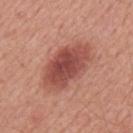This is a white-light tile.
A lesion tile, about 15 mm wide, cut from a 3D total-body photograph.
From the mid back.
The recorded lesion diameter is about 7 mm.
A male subject, aged 63–67.